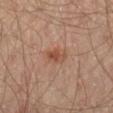This lesion was catalogued during total-body skin photography and was not selected for biopsy. The patient is a male aged approximately 65. The total-body-photography lesion software estimated a border-irregularity rating of about 3/10, a within-lesion color-variation index near 1.5/10, and radial color variation of about 0.5. And it measured a classifier nevus-likeness of about 70/100. This is a cross-polarized tile. The lesion's longest dimension is about 3 mm. A 15 mm close-up tile from a total-body photography series done for melanoma screening. From the left thigh.